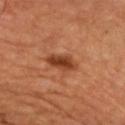Findings:
– notes: total-body-photography surveillance lesion; no biopsy
– body site: the upper back
– patient: male, aged 63 to 67
– image source: total-body-photography crop, ~15 mm field of view
– illumination: cross-polarized
– diameter: ≈4 mm